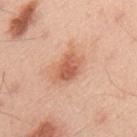Q: Is there a histopathology result?
A: imaged on a skin check; not biopsied
Q: What is the imaging modality?
A: 15 mm crop, total-body photography
Q: What are the patient's age and sex?
A: male, aged approximately 45
Q: What is the lesion's diameter?
A: about 4 mm
Q: Illumination type?
A: white-light
Q: What is the anatomic site?
A: the mid back